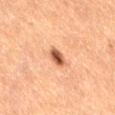  biopsy_status: not biopsied; imaged during a skin examination
  patient:
    sex: female
    age_approx: 60
  lighting: cross-polarized
  site: right thigh
  image:
    source: total-body photography crop
    field_of_view_mm: 15